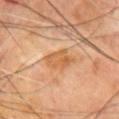  biopsy_status: not biopsied; imaged during a skin examination
  image:
    source: total-body photography crop
    field_of_view_mm: 15
  patient:
    sex: male
    age_approx: 70
  site: front of the torso